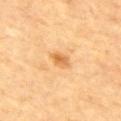Clinical impression: No biopsy was performed on this lesion — it was imaged during a full skin examination and was not determined to be concerning. Background: A male patient, about 85 years old or older. On the chest. A region of skin cropped from a whole-body photographic capture, roughly 15 mm wide.